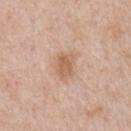{"biopsy_status": "not biopsied; imaged during a skin examination", "automated_metrics": {"peripheral_color_asymmetry": 1.0, "nevus_likeness_0_100": 40, "lesion_detection_confidence_0_100": 100}, "patient": {"sex": "male", "age_approx": 60}, "site": "chest", "lighting": "white-light", "image": {"source": "total-body photography crop", "field_of_view_mm": 15}}